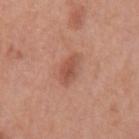No biopsy was performed on this lesion — it was imaged during a full skin examination and was not determined to be concerning. Automated image analysis of the tile measured an area of roughly 5.5 mm² and an outline eccentricity of about 0.8 (0 = round, 1 = elongated). It also reported border irregularity of about 2 on a 0–10 scale, a color-variation rating of about 2.5/10, and radial color variation of about 1. The software also gave an automated nevus-likeness rating near 55 out of 100 and lesion-presence confidence of about 100/100. Imaged with white-light lighting. A male subject aged approximately 70. The lesion is located on the mid back. A roughly 15 mm field-of-view crop from a total-body skin photograph. Measured at roughly 3.5 mm in maximum diameter.